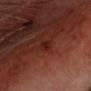Impression:
The lesion was tiled from a total-body skin photograph and was not biopsied.
Clinical summary:
Measured at roughly 2.5 mm in maximum diameter. The tile uses cross-polarized illumination. A close-up tile cropped from a whole-body skin photograph, about 15 mm across. The patient is a male aged 63 to 67.